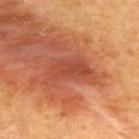Q: Was this lesion biopsied?
A: imaged on a skin check; not biopsied
Q: Lesion location?
A: the upper back
Q: Patient demographics?
A: male, aged 48 to 52
Q: Illumination type?
A: cross-polarized
Q: How large is the lesion?
A: ≈5 mm
Q: What kind of image is this?
A: ~15 mm crop, total-body skin-cancer survey